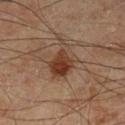Part of a total-body skin-imaging series; this lesion was reviewed on a skin check and was not flagged for biopsy. A male patient, approximately 70 years of age. From the left lower leg. The lesion's longest dimension is about 5 mm. A region of skin cropped from a whole-body photographic capture, roughly 15 mm wide.